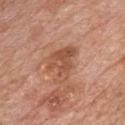Clinical impression: Imaged during a routine full-body skin examination; the lesion was not biopsied and no histopathology is available. Context: Located on the front of the torso. About 4.5 mm across. A male subject in their 60s. This is a white-light tile. A close-up tile cropped from a whole-body skin photograph, about 15 mm across.